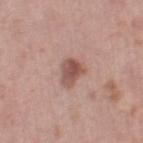{"biopsy_status": "not biopsied; imaged during a skin examination", "site": "right thigh", "image": {"source": "total-body photography crop", "field_of_view_mm": 15}, "patient": {"sex": "male", "age_approx": 55}}